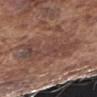  biopsy_status: not biopsied; imaged during a skin examination
  lighting: white-light
  patient:
    sex: male
    age_approx: 75
  lesion_size:
    long_diameter_mm_approx: 7.0
  site: right upper arm
  image:
    source: total-body photography crop
    field_of_view_mm: 15